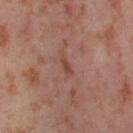Clinical impression: The lesion was photographed on a routine skin check and not biopsied; there is no pathology result. Context: Approximately 3 mm at its widest. Automated tile analysis of the lesion measured internal color variation of about 0 on a 0–10 scale. It also reported an automated nevus-likeness rating near 0 out of 100 and a lesion-detection confidence of about 85/100. The tile uses cross-polarized illumination. The lesion is located on the left thigh. A lesion tile, about 15 mm wide, cut from a 3D total-body photograph. A female subject aged approximately 55.